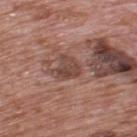Impression: This lesion was catalogued during total-body skin photography and was not selected for biopsy. Background: The patient is a male about 70 years old. The lesion is on the upper back. Cropped from a total-body skin-imaging series; the visible field is about 15 mm. Captured under white-light illumination. Measured at roughly 3 mm in maximum diameter.